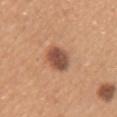workup — no biopsy performed (imaged during a skin exam)
size — about 3 mm
lighting — white-light
anatomic site — the right upper arm
subject — female, about 30 years old
image source — ~15 mm crop, total-body skin-cancer survey